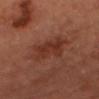{"biopsy_status": "not biopsied; imaged during a skin examination", "patient": {"sex": "male", "age_approx": 65}, "site": "head or neck", "image": {"source": "total-body photography crop", "field_of_view_mm": 15}, "lesion_size": {"long_diameter_mm_approx": 5.0}}